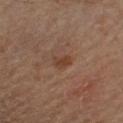Clinical impression:
The lesion was photographed on a routine skin check and not biopsied; there is no pathology result.
Acquisition and patient details:
Located on the left upper arm. A male subject aged 53 to 57. A roughly 15 mm field-of-view crop from a total-body skin photograph. Captured under cross-polarized illumination.